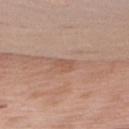Recorded during total-body skin imaging; not selected for excision or biopsy. A region of skin cropped from a whole-body photographic capture, roughly 15 mm wide. From the chest. The subject is a female aged approximately 70.